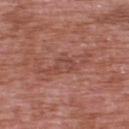A male patient aged around 70.
A roughly 15 mm field-of-view crop from a total-body skin photograph.
This is a white-light tile.
The lesion is located on the upper back.